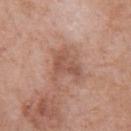No biopsy was performed on this lesion — it was imaged during a full skin examination and was not determined to be concerning. A male patient, in their 70s. Approximately 4 mm at its widest. A roughly 15 mm field-of-view crop from a total-body skin photograph. Located on the front of the torso.